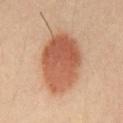Q: Was a biopsy performed?
A: imaged on a skin check; not biopsied
Q: How was this image acquired?
A: ~15 mm tile from a whole-body skin photo
Q: Lesion location?
A: the front of the torso
Q: Who is the patient?
A: male, in their 40s
Q: Lesion size?
A: ~8 mm (longest diameter)
Q: How was the tile lit?
A: cross-polarized illumination